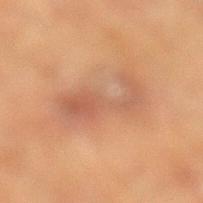The patient is a male in their mid- to late 80s or older.
A close-up tile cropped from a whole-body skin photograph, about 15 mm across.
The lesion is located on the left lower leg.
Automated image analysis of the tile measured a footprint of about 14 mm², an eccentricity of roughly 0.9, and a shape-asymmetry score of about 0.4 (0 = symmetric).
About 6.5 mm across.